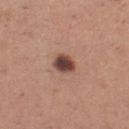follow-up = catalogued during a skin exam; not biopsied.